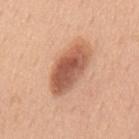Notes:
– follow-up — catalogued during a skin exam; not biopsied
– lighting — white-light
– body site — the mid back
– automated lesion analysis — border irregularity of about 2 on a 0–10 scale, a within-lesion color-variation index near 6.5/10, and a peripheral color-asymmetry measure near 2; a nevus-likeness score of about 70/100
– imaging modality — ~15 mm tile from a whole-body skin photo
– lesion diameter — ≈6.5 mm
– subject — male, approximately 50 years of age